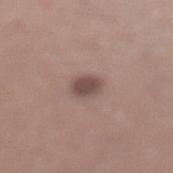Captured during whole-body skin photography for melanoma surveillance; the lesion was not biopsied. A roughly 15 mm field-of-view crop from a total-body skin photograph. A male subject, in their 40s. The lesion is located on the left lower leg. Automated tile analysis of the lesion measured an area of roughly 5.5 mm², an outline eccentricity of about 0.55 (0 = round, 1 = elongated), and a symmetry-axis asymmetry near 0.2. And it measured a lesion color around L≈48 a*≈16 b*≈20 in CIELAB, roughly 11 lightness units darker than nearby skin, and a normalized lesion–skin contrast near 8.5. It also reported a detector confidence of about 100 out of 100 that the crop contains a lesion. The tile uses white-light illumination. Approximately 3 mm at its widest.